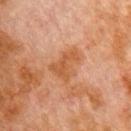biopsy status: imaged on a skin check; not biopsied
acquisition: ~15 mm tile from a whole-body skin photo
lighting: cross-polarized
subject: male, about 80 years old
TBP lesion metrics: a footprint of about 8 mm² and two-axis asymmetry of about 0.45; about 6 CIELAB-L* units darker than the surrounding skin and a lesion-to-skin contrast of about 6 (normalized; higher = more distinct); a border-irregularity index near 5/10 and peripheral color asymmetry of about 0.5; a nevus-likeness score of about 0/100 and a lesion-detection confidence of about 100/100
body site: the chest